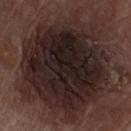Clinical impression: Part of a total-body skin-imaging series; this lesion was reviewed on a skin check and was not flagged for biopsy. Context: Located on the chest. A male patient aged around 80. The tile uses white-light illumination. A 15 mm crop from a total-body photograph taken for skin-cancer surveillance. Automated image analysis of the tile measured a lesion color around L≈22 a*≈14 b*≈16 in CIELAB and roughly 12 lightness units darker than nearby skin. It also reported a border-irregularity rating of about 3.5/10, a within-lesion color-variation index near 6.5/10, and a peripheral color-asymmetry measure near 1.5. It also reported a nevus-likeness score of about 10/100.